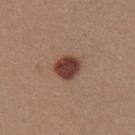Image and clinical context:
This is a white-light tile. The lesion is located on the chest. Cropped from a whole-body photographic skin survey; the tile spans about 15 mm. The patient is a female in their 30s. Automated tile analysis of the lesion measured internal color variation of about 3 on a 0–10 scale and peripheral color asymmetry of about 1.